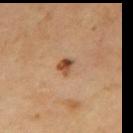lighting: cross-polarized illumination | anatomic site: the upper back | subject: male, roughly 70 years of age | image: ~15 mm tile from a whole-body skin photo | diameter: ≈2 mm.